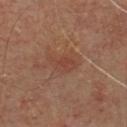Clinical impression: Imaged during a routine full-body skin examination; the lesion was not biopsied and no histopathology is available. Background: Captured under cross-polarized illumination. A male subject aged around 65. A 15 mm crop from a total-body photograph taken for skin-cancer surveillance. From the chest. The recorded lesion diameter is about 3.5 mm. Automated image analysis of the tile measured an eccentricity of roughly 0.8.